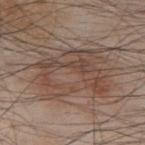– workup: no biopsy performed (imaged during a skin exam)
– automated lesion analysis: an average lesion color of about L≈47 a*≈16 b*≈24 (CIELAB) and a lesion-to-skin contrast of about 6 (normalized; higher = more distinct)
– subject: male, roughly 45 years of age
– illumination: white-light illumination
– site: the back
– size: ≈9 mm
– image: 15 mm crop, total-body photography